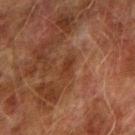No biopsy was performed on this lesion — it was imaged during a full skin examination and was not determined to be concerning. The recorded lesion diameter is about 3 mm. This image is a 15 mm lesion crop taken from a total-body photograph. Captured under cross-polarized illumination. From the right upper arm. The lesion-visualizer software estimated an eccentricity of roughly 0.85 and a symmetry-axis asymmetry near 0.3. The analysis additionally found border irregularity of about 3 on a 0–10 scale, internal color variation of about 2 on a 0–10 scale, and peripheral color asymmetry of about 0.5. The software also gave a classifier nevus-likeness of about 0/100 and a lesion-detection confidence of about 95/100. A male subject, aged approximately 75.